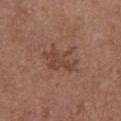| key | value |
|---|---|
| notes | catalogued during a skin exam; not biopsied |
| body site | the chest |
| patient | female, in their mid-60s |
| lighting | white-light illumination |
| lesion size | ≈4 mm |
| imaging modality | ~15 mm tile from a whole-body skin photo |